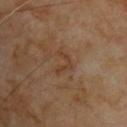* tile lighting · cross-polarized
* acquisition · ~15 mm crop, total-body skin-cancer survey
* body site · the upper back
* subject · male, approximately 70 years of age